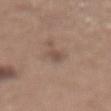<tbp_lesion>
<biopsy_status>not biopsied; imaged during a skin examination</biopsy_status>
<lighting>white-light</lighting>
<automated_metrics>
  <nevus_likeness_0_100>0</nevus_likeness_0_100>
</automated_metrics>
<image>
  <source>total-body photography crop</source>
  <field_of_view_mm>15</field_of_view_mm>
</image>
<lesion_size>
  <long_diameter_mm_approx>2.5</long_diameter_mm_approx>
</lesion_size>
<patient>
  <sex>female</sex>
  <age_approx>65</age_approx>
</patient>
<site>abdomen</site>
</tbp_lesion>